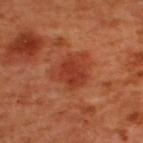notes: no biopsy performed (imaged during a skin exam); patient: male, about 50 years old; anatomic site: the upper back; image: ~15 mm tile from a whole-body skin photo.